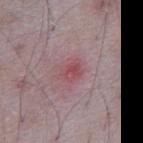Case summary:
- subject: male, aged 63 to 67
- body site: the front of the torso
- acquisition: ~15 mm tile from a whole-body skin photo
- size: about 3 mm
- lighting: white-light illumination